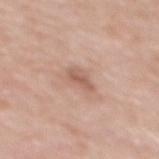follow-up = no biopsy performed (imaged during a skin exam)
imaging modality = 15 mm crop, total-body photography
lesion diameter = ≈3 mm
patient = female, in their 40s
site = the mid back
TBP lesion metrics = a border-irregularity rating of about 3/10 and internal color variation of about 2.5 on a 0–10 scale
lighting = white-light illumination